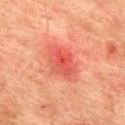automated lesion analysis = an area of roughly 10 mm² and an eccentricity of roughly 0.75; a mean CIELAB color near L≈48 a*≈35 b*≈31, roughly 8 lightness units darker than nearby skin, and a normalized border contrast of about 6; a border-irregularity index near 1.5/10, a within-lesion color-variation index near 6/10, and peripheral color asymmetry of about 2
tile lighting = cross-polarized illumination
subject = male, aged around 75
size = ≈4.5 mm
image = ~15 mm crop, total-body skin-cancer survey
location = the mid back